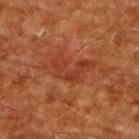Notes:
– biopsy status: catalogued during a skin exam; not biopsied
– lesion size: about 5.5 mm
– imaging modality: total-body-photography crop, ~15 mm field of view
– site: the upper back
– illumination: cross-polarized illumination
– patient: male, aged approximately 60